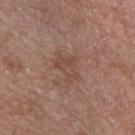Part of a total-body skin-imaging series; this lesion was reviewed on a skin check and was not flagged for biopsy. An algorithmic analysis of the crop reported an area of roughly 6 mm², an outline eccentricity of about 0.75 (0 = round, 1 = elongated), and a symmetry-axis asymmetry near 0.45. The analysis additionally found an average lesion color of about L≈46 a*≈19 b*≈27 (CIELAB), roughly 6 lightness units darker than nearby skin, and a normalized border contrast of about 5. It also reported a border-irregularity index near 5/10, a within-lesion color-variation index near 2.5/10, and a peripheral color-asymmetry measure near 1. It also reported an automated nevus-likeness rating near 0 out of 100 and a lesion-detection confidence of about 100/100. A male patient, aged 48–52. Approximately 3.5 mm at its widest. A 15 mm close-up extracted from a 3D total-body photography capture. The lesion is on the chest. Imaged with white-light lighting.